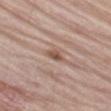<record>
<biopsy_status>not biopsied; imaged during a skin examination</biopsy_status>
<patient>
  <sex>male</sex>
  <age_approx>80</age_approx>
</patient>
<image>
  <source>total-body photography crop</source>
  <field_of_view_mm>15</field_of_view_mm>
</image>
<lighting>white-light</lighting>
<automated_metrics>
  <color_variation_0_10>3.5</color_variation_0_10>
  <peripheral_color_asymmetry>1.5</peripheral_color_asymmetry>
</automated_metrics>
<site>leg</site>
<lesion_size>
  <long_diameter_mm_approx>3.0</long_diameter_mm_approx>
</lesion_size>
</record>